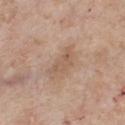The lesion was tiled from a total-body skin photograph and was not biopsied.
The total-body-photography lesion software estimated a nevus-likeness score of about 0/100 and lesion-presence confidence of about 100/100.
Imaged with white-light lighting.
The subject is a male aged 53 to 57.
From the chest.
Cropped from a total-body skin-imaging series; the visible field is about 15 mm.